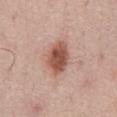notes: catalogued during a skin exam; not biopsied
automated metrics: a footprint of about 9.5 mm², an outline eccentricity of about 0.75 (0 = round, 1 = elongated), and a shape-asymmetry score of about 0.15 (0 = symmetric); a lesion–skin lightness drop of about 15 and a normalized lesion–skin contrast near 10; border irregularity of about 2 on a 0–10 scale, a within-lesion color-variation index near 3.5/10, and peripheral color asymmetry of about 1; an automated nevus-likeness rating near 95 out of 100 and a lesion-detection confidence of about 100/100
image: total-body-photography crop, ~15 mm field of view
subject: male, roughly 60 years of age
location: the front of the torso
illumination: white-light